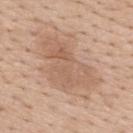Imaged during a routine full-body skin examination; the lesion was not biopsied and no histopathology is available.
Longest diameter approximately 8 mm.
From the mid back.
The subject is a male about 60 years old.
This is a white-light tile.
A 15 mm crop from a total-body photograph taken for skin-cancer surveillance.